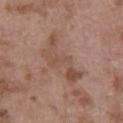Q: Was this lesion biopsied?
A: total-body-photography surveillance lesion; no biopsy
Q: How was this image acquired?
A: 15 mm crop, total-body photography
Q: What is the lesion's diameter?
A: ≈7 mm
Q: Who is the patient?
A: male, aged 53 to 57
Q: Where on the body is the lesion?
A: the lower back